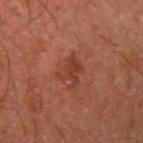The lesion was photographed on a routine skin check and not biopsied; there is no pathology result. A lesion tile, about 15 mm wide, cut from a 3D total-body photograph. About 3 mm across. The lesion is located on the right upper arm. An algorithmic analysis of the crop reported an area of roughly 6 mm² and an eccentricity of roughly 0.6. The analysis additionally found an average lesion color of about L≈30 a*≈21 b*≈25 (CIELAB) and a normalized lesion–skin contrast near 6. And it measured a nevus-likeness score of about 0/100 and a detector confidence of about 100 out of 100 that the crop contains a lesion. The tile uses cross-polarized illumination. A male patient aged approximately 50.